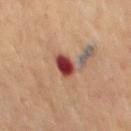The lesion was photographed on a routine skin check and not biopsied; there is no pathology result.